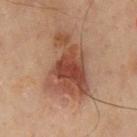The lesion was photographed on a routine skin check and not biopsied; there is no pathology result.
Captured under cross-polarized illumination.
Cropped from a total-body skin-imaging series; the visible field is about 15 mm.
A male patient about 70 years old.
Approximately 9 mm at its widest.
On the front of the torso.